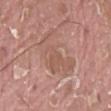Recorded during total-body skin imaging; not selected for excision or biopsy. A male patient about 40 years old. Cropped from a total-body skin-imaging series; the visible field is about 15 mm. The lesion is located on the left thigh. This is a white-light tile. About 6 mm across.